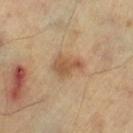No biopsy was performed on this lesion — it was imaged during a full skin examination and was not determined to be concerning. The lesion is on the right lower leg. A 15 mm close-up tile from a total-body photography series done for melanoma screening. A subject aged 58–62.